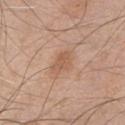Recorded during total-body skin imaging; not selected for excision or biopsy. A roughly 15 mm field-of-view crop from a total-body skin photograph. The lesion is located on the front of the torso. The patient is a male in their mid- to late 40s.